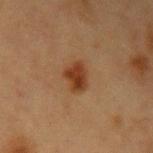  biopsy_status: not biopsied; imaged during a skin examination
  lighting: cross-polarized
  patient:
    sex: female
    age_approx: 40
  image:
    source: total-body photography crop
    field_of_view_mm: 15
  automated_metrics:
    area_mm2_approx: 6.0
    eccentricity: 0.6
    shape_asymmetry: 0.25
    nevus_likeness_0_100: 100
    lesion_detection_confidence_0_100: 100
  site: arm
  lesion_size:
    long_diameter_mm_approx: 3.0